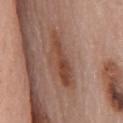<tbp_lesion>
  <image>
    <source>total-body photography crop</source>
    <field_of_view_mm>15</field_of_view_mm>
  </image>
  <patient>
    <sex>male</sex>
    <age_approx>80</age_approx>
  </patient>
  <site>chest</site>
  <lighting>white-light</lighting>
  <automated_metrics>
    <area_mm2_approx>11.0</area_mm2_approx>
    <eccentricity>0.95</eccentricity>
    <shape_asymmetry>0.3</shape_asymmetry>
    <cielab_L>46</cielab_L>
    <cielab_a>22</cielab_a>
    <cielab_b>29</cielab_b>
    <vs_skin_darker_L>9.0</vs_skin_darker_L>
    <vs_skin_contrast_norm>7.5</vs_skin_contrast_norm>
    <border_irregularity_0_10>5.0</border_irregularity_0_10>
    <color_variation_0_10>5.0</color_variation_0_10>
    <peripheral_color_asymmetry>2.0</peripheral_color_asymmetry>
  </automated_metrics>
  <lesion_size>
    <long_diameter_mm_approx>6.5</long_diameter_mm_approx>
  </lesion_size>
</tbp_lesion>